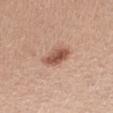Assessment: The lesion was photographed on a routine skin check and not biopsied; there is no pathology result. Clinical summary: The patient is a female approximately 55 years of age. A 15 mm close-up extracted from a 3D total-body photography capture. The lesion is located on the right thigh. Approximately 3.5 mm at its widest. This is a white-light tile.